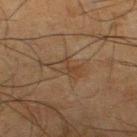  biopsy_status: not biopsied; imaged during a skin examination
  site: left thigh
  patient:
    sex: male
    age_approx: 65
  image:
    source: total-body photography crop
    field_of_view_mm: 15
  lesion_size:
    long_diameter_mm_approx: 4.0
  automated_metrics:
    border_irregularity_0_10: 4.0
    peripheral_color_asymmetry: 1.5